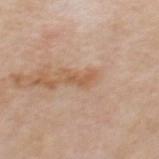Findings:
– site · the mid back
– image · ~15 mm tile from a whole-body skin photo
– patient · male, in their mid- to late 60s
– image-analysis metrics · an area of roughly 3 mm², an outline eccentricity of about 0.9 (0 = round, 1 = elongated), and two-axis asymmetry of about 0.4; an average lesion color of about L≈57 a*≈20 b*≈34 (CIELAB) and about 8 CIELAB-L* units darker than the surrounding skin; a within-lesion color-variation index near 0/10 and peripheral color asymmetry of about 0; an automated nevus-likeness rating near 5 out of 100 and a detector confidence of about 100 out of 100 that the crop contains a lesion
– lesion size · about 3 mm
– illumination · white-light